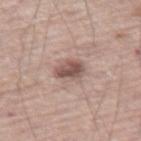{"biopsy_status": "not biopsied; imaged during a skin examination", "site": "leg", "lesion_size": {"long_diameter_mm_approx": 3.0}, "automated_metrics": {"nevus_likeness_0_100": 75, "lesion_detection_confidence_0_100": 100}, "image": {"source": "total-body photography crop", "field_of_view_mm": 15}, "lighting": "white-light", "patient": {"sex": "male", "age_approx": 70}}